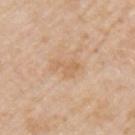The recorded lesion diameter is about 3.5 mm. Located on the left upper arm. This is a white-light tile. Cropped from a total-body skin-imaging series; the visible field is about 15 mm. A male subject, aged 73–77.